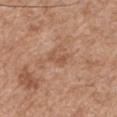notes: catalogued during a skin exam; not biopsied
subject: male, aged 53–57
image-analysis metrics: a lesion area of about 2.5 mm², an eccentricity of roughly 0.9, and a shape-asymmetry score of about 0.4 (0 = symmetric)
anatomic site: the right upper arm
lesion size: ≈2.5 mm
lighting: white-light
image: total-body-photography crop, ~15 mm field of view